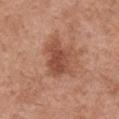Clinical impression:
The lesion was photographed on a routine skin check and not biopsied; there is no pathology result.
Acquisition and patient details:
A region of skin cropped from a whole-body photographic capture, roughly 15 mm wide. On the front of the torso. This is a white-light tile. Measured at roughly 5 mm in maximum diameter. A female patient, aged 63–67. The lesion-visualizer software estimated a lesion area of about 13 mm² and two-axis asymmetry of about 0.3.